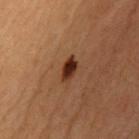Assessment:
Part of a total-body skin-imaging series; this lesion was reviewed on a skin check and was not flagged for biopsy.
Clinical summary:
Automated tile analysis of the lesion measured a lesion area of about 4.5 mm², a shape eccentricity near 0.85, and a symmetry-axis asymmetry near 0.3. And it measured a border-irregularity rating of about 3/10, a color-variation rating of about 3.5/10, and a peripheral color-asymmetry measure near 1. From the chest. The patient is a male aged 48 to 52. Longest diameter approximately 3 mm. Cropped from a whole-body photographic skin survey; the tile spans about 15 mm. This is a cross-polarized tile.